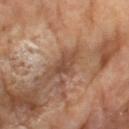Q: Is there a histopathology result?
A: total-body-photography surveillance lesion; no biopsy
Q: Who is the patient?
A: male, aged approximately 65
Q: How was this image acquired?
A: total-body-photography crop, ~15 mm field of view